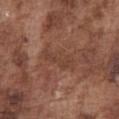Findings:
- follow-up: imaged on a skin check; not biopsied
- tile lighting: white-light
- image: ~15 mm crop, total-body skin-cancer survey
- body site: the chest
- subject: male, roughly 75 years of age
- lesion diameter: about 4.5 mm